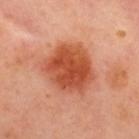Part of a total-body skin-imaging series; this lesion was reviewed on a skin check and was not flagged for biopsy. From the upper back. Cropped from a total-body skin-imaging series; the visible field is about 15 mm. A male subject in their 50s.